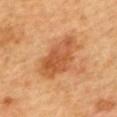Captured during whole-body skin photography for melanoma surveillance; the lesion was not biopsied.
Longest diameter approximately 6.5 mm.
A close-up tile cropped from a whole-body skin photograph, about 15 mm across.
Located on the upper back.
A female subject aged 58 to 62.